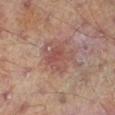No biopsy was performed on this lesion — it was imaged during a full skin examination and was not determined to be concerning. A region of skin cropped from a whole-body photographic capture, roughly 15 mm wide. Imaged with cross-polarized lighting. On the leg. The recorded lesion diameter is about 4 mm. Automated tile analysis of the lesion measured a border-irregularity index near 4.5/10. And it measured a nevus-likeness score of about 5/100 and lesion-presence confidence of about 100/100. The patient is a male roughly 70 years of age.